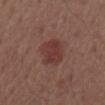This lesion was catalogued during total-body skin photography and was not selected for biopsy.
The lesion is on the abdomen.
The subject is a male in their mid-50s.
This is a white-light tile.
Approximately 3.5 mm at its widest.
A close-up tile cropped from a whole-body skin photograph, about 15 mm across.